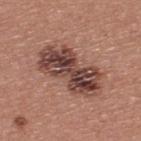Impression:
The lesion was photographed on a routine skin check and not biopsied; there is no pathology result.
Clinical summary:
This is a white-light tile. Located on the upper back. Measured at roughly 8 mm in maximum diameter. A lesion tile, about 15 mm wide, cut from a 3D total-body photograph. Automated tile analysis of the lesion measured a footprint of about 23 mm², a shape eccentricity near 0.9, and a symmetry-axis asymmetry near 0.25. A male subject, approximately 40 years of age.